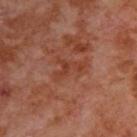Findings:
* notes: imaged on a skin check; not biopsied
* image source: total-body-photography crop, ~15 mm field of view
* site: the upper back
* patient: male, roughly 70 years of age